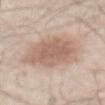Assessment:
The lesion was tiled from a total-body skin photograph and was not biopsied.
Context:
From the abdomen. Captured under white-light illumination. About 7 mm across. A 15 mm crop from a total-body photograph taken for skin-cancer surveillance. A male patient aged approximately 70. The lesion-visualizer software estimated about 11 CIELAB-L* units darker than the surrounding skin and a normalized border contrast of about 7. The software also gave an automated nevus-likeness rating near 80 out of 100 and a detector confidence of about 100 out of 100 that the crop contains a lesion.